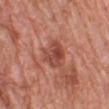The lesion was tiled from a total-body skin photograph and was not biopsied.